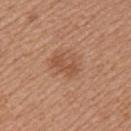Notes:
* follow-up: total-body-photography surveillance lesion; no biopsy
* automated metrics: an automated nevus-likeness rating near 35 out of 100 and lesion-presence confidence of about 100/100
* subject: female, in their mid-30s
* anatomic site: the left upper arm
* illumination: white-light
* acquisition: 15 mm crop, total-body photography
* size: ~3.5 mm (longest diameter)